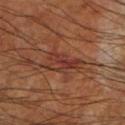Imaged during a routine full-body skin examination; the lesion was not biopsied and no histopathology is available.
A close-up tile cropped from a whole-body skin photograph, about 15 mm across.
Longest diameter approximately 4.5 mm.
From the left lower leg.
Captured under cross-polarized illumination.
The patient is a male aged approximately 60.